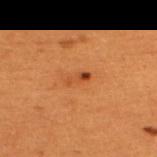Assessment: Captured during whole-body skin photography for melanoma surveillance; the lesion was not biopsied. Image and clinical context: On the upper back. Cropped from a total-body skin-imaging series; the visible field is about 15 mm. Measured at roughly 2.5 mm in maximum diameter. The tile uses cross-polarized illumination. The patient is a male about 50 years old.